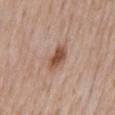Clinical impression: No biopsy was performed on this lesion — it was imaged during a full skin examination and was not determined to be concerning. Image and clinical context: A lesion tile, about 15 mm wide, cut from a 3D total-body photograph. On the mid back. The subject is a male aged around 75. Approximately 4 mm at its widest.